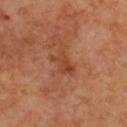The lesion was photographed on a routine skin check and not biopsied; there is no pathology result.
On the upper back.
The subject is a female aged approximately 40.
A roughly 15 mm field-of-view crop from a total-body skin photograph.
Approximately 3.5 mm at its widest.
Imaged with cross-polarized lighting.